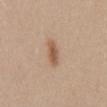Impression: Part of a total-body skin-imaging series; this lesion was reviewed on a skin check and was not flagged for biopsy. Background: A 15 mm close-up extracted from a 3D total-body photography capture. The lesion is on the mid back. The total-body-photography lesion software estimated a lesion area of about 5 mm², an eccentricity of roughly 0.95, and a shape-asymmetry score of about 0.2 (0 = symmetric). It also reported a border-irregularity index near 3/10 and a within-lesion color-variation index near 2.5/10. About 4 mm across. A female patient approximately 40 years of age. This is a white-light tile.